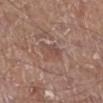Notes:
* notes — no biopsy performed (imaged during a skin exam)
* body site — the right lower leg
* image — 15 mm crop, total-body photography
* subject — male, aged around 70